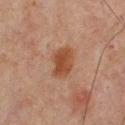Clinical impression:
This lesion was catalogued during total-body skin photography and was not selected for biopsy.
Context:
The lesion-visualizer software estimated an area of roughly 9 mm², a shape eccentricity near 0.7, and a symmetry-axis asymmetry near 0.2. The software also gave a within-lesion color-variation index near 2.5/10 and a peripheral color-asymmetry measure near 0.5. Captured under cross-polarized illumination. About 4 mm across. Cropped from a total-body skin-imaging series; the visible field is about 15 mm. The subject is a male roughly 65 years of age. The lesion is located on the upper back.